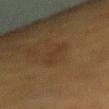workup: total-body-photography surveillance lesion; no biopsy | site: the arm | illumination: cross-polarized | subject: female, aged approximately 55 | lesion diameter: ≈3.5 mm | image source: total-body-photography crop, ~15 mm field of view.